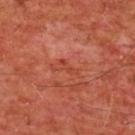  biopsy_status: not biopsied; imaged during a skin examination
  image:
    source: total-body photography crop
    field_of_view_mm: 15
  patient:
    sex: male
    age_approx: 60
  site: chest